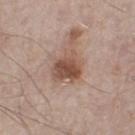biopsy status: no biopsy performed (imaged during a skin exam); site: the leg; subject: male, in their mid- to late 50s; image: 15 mm crop, total-body photography.